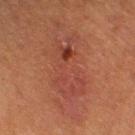workup = total-body-photography surveillance lesion; no biopsy
location = the right lower leg
lighting = cross-polarized
lesion diameter = about 7.5 mm
subject = male, aged 63 to 67
automated metrics = an area of roughly 17 mm²; about 5 CIELAB-L* units darker than the surrounding skin and a normalized border contrast of about 5.5; a peripheral color-asymmetry measure near 2.5
image = ~15 mm tile from a whole-body skin photo